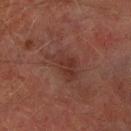| feature | finding |
|---|---|
| workup | imaged on a skin check; not biopsied |
| size | ≈4.5 mm |
| automated lesion analysis | a lesion area of about 8 mm² and a shape-asymmetry score of about 0.4 (0 = symmetric); a lesion–skin lightness drop of about 5 and a lesion-to-skin contrast of about 6 (normalized; higher = more distinct); a border-irregularity rating of about 5/10, a color-variation rating of about 2.5/10, and peripheral color asymmetry of about 1; a nevus-likeness score of about 0/100 |
| anatomic site | the left forearm |
| subject | male, aged 73–77 |
| imaging modality | ~15 mm tile from a whole-body skin photo |
| illumination | cross-polarized |